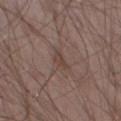  biopsy_status: not biopsied; imaged during a skin examination
  site: left thigh
  image:
    source: total-body photography crop
    field_of_view_mm: 15
  lighting: white-light
  patient:
    sex: male
    age_approx: 65
  lesion_size:
    long_diameter_mm_approx: 2.5